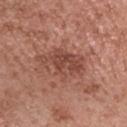workup: catalogued during a skin exam; not biopsied | anatomic site: the head or neck | lesion diameter: about 6 mm | illumination: white-light illumination | imaging modality: total-body-photography crop, ~15 mm field of view | patient: female, in their mid-50s.